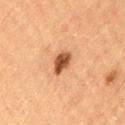Q: Was a biopsy performed?
A: imaged on a skin check; not biopsied
Q: How was this image acquired?
A: ~15 mm crop, total-body skin-cancer survey
Q: Where on the body is the lesion?
A: the left thigh
Q: How large is the lesion?
A: about 3.5 mm
Q: What are the patient's age and sex?
A: female, aged around 60
Q: What did automated image analysis measure?
A: an area of roughly 5 mm² and a symmetry-axis asymmetry near 0.2; a border-irregularity rating of about 2/10 and radial color variation of about 1.5